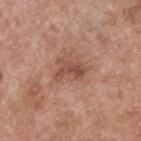notes: no biopsy performed (imaged during a skin exam) | patient: male, aged 53 to 57 | body site: the upper back | size: ≈3.5 mm | lighting: white-light illumination | acquisition: total-body-photography crop, ~15 mm field of view.